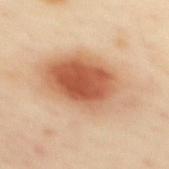Clinical impression: Part of a total-body skin-imaging series; this lesion was reviewed on a skin check and was not flagged for biopsy. Background: This is a cross-polarized tile. A lesion tile, about 15 mm wide, cut from a 3D total-body photograph. The lesion's longest dimension is about 9.5 mm. The subject is a female approximately 40 years of age. Located on the upper back.